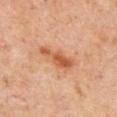Assessment:
The lesion was photographed on a routine skin check and not biopsied; there is no pathology result.
Image and clinical context:
Measured at roughly 4.5 mm in maximum diameter. The patient is a male approximately 55 years of age. A close-up tile cropped from a whole-body skin photograph, about 15 mm across. Located on the front of the torso.